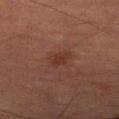The lesion was tiled from a total-body skin photograph and was not biopsied.
A male patient, in their mid- to late 80s or older.
A lesion tile, about 15 mm wide, cut from a 3D total-body photograph.
The recorded lesion diameter is about 2.5 mm.
The lesion is located on the right lower leg.
The lesion-visualizer software estimated an eccentricity of roughly 0.7 and a symmetry-axis asymmetry near 0.25. It also reported a lesion–skin lightness drop of about 5 and a normalized lesion–skin contrast near 6.5. It also reported a border-irregularity index near 2.5/10, a within-lesion color-variation index near 1.5/10, and peripheral color asymmetry of about 0.5. And it measured a nevus-likeness score of about 30/100 and lesion-presence confidence of about 100/100.